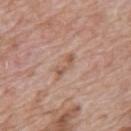No biopsy was performed on this lesion — it was imaged during a full skin examination and was not determined to be concerning. The lesion is on the mid back. Automated tile analysis of the lesion measured a color-variation rating of about 0/10 and radial color variation of about 0. A region of skin cropped from a whole-body photographic capture, roughly 15 mm wide. A male subject, approximately 70 years of age.